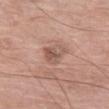This lesion was catalogued during total-body skin photography and was not selected for biopsy. The subject is a female aged approximately 75. The lesion's longest dimension is about 3.5 mm. A 15 mm crop from a total-body photograph taken for skin-cancer surveillance. This is a white-light tile. From the left thigh.